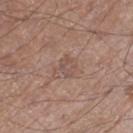biopsy_status: not biopsied; imaged during a skin examination
patient:
  sex: male
  age_approx: 60
lesion_size:
  long_diameter_mm_approx: 2.5
site: left thigh
lighting: white-light
image:
  source: total-body photography crop
  field_of_view_mm: 15
automated_metrics:
  cielab_L: 50
  cielab_a: 17
  cielab_b: 23
  vs_skin_darker_L: 7.0
  vs_skin_contrast_norm: 5.0
  color_variation_0_10: 0.0
  peripheral_color_asymmetry: 0.0
  nevus_likeness_0_100: 0
  lesion_detection_confidence_0_100: 100